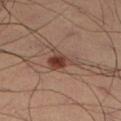No biopsy was performed on this lesion — it was imaged during a full skin examination and was not determined to be concerning.
Measured at roughly 4 mm in maximum diameter.
Captured under cross-polarized illumination.
A male subject, roughly 40 years of age.
From the right lower leg.
Cropped from a total-body skin-imaging series; the visible field is about 15 mm.